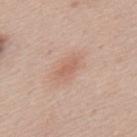biopsy status: catalogued during a skin exam; not biopsied | location: the mid back | subject: male, in their mid-40s | imaging modality: 15 mm crop, total-body photography | TBP lesion metrics: a lesion–skin lightness drop of about 7 and a lesion-to-skin contrast of about 5 (normalized; higher = more distinct) | diameter: ~2.5 mm (longest diameter).